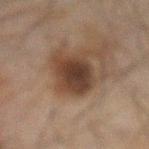The tile uses cross-polarized illumination.
A region of skin cropped from a whole-body photographic capture, roughly 15 mm wide.
A male subject, aged around 60.
About 5 mm across.
Located on the front of the torso.